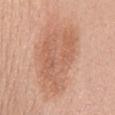follow-up = imaged on a skin check; not biopsied | lesion size = ~9 mm (longest diameter) | subject = female, aged around 40 | acquisition = ~15 mm crop, total-body skin-cancer survey | illumination = white-light | body site = the back | automated lesion analysis = a footprint of about 31 mm² and a shape eccentricity near 0.85; border irregularity of about 4 on a 0–10 scale and a color-variation rating of about 3.5/10.